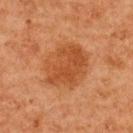| field | value |
|---|---|
| notes | catalogued during a skin exam; not biopsied |
| location | the back |
| patient | female, about 50 years old |
| tile lighting | cross-polarized illumination |
| imaging modality | total-body-photography crop, ~15 mm field of view |
| size | ~6 mm (longest diameter) |